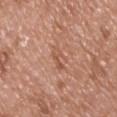Q: Was a biopsy performed?
A: total-body-photography surveillance lesion; no biopsy
Q: How was this image acquired?
A: ~15 mm crop, total-body skin-cancer survey
Q: Who is the patient?
A: male, aged 48–52
Q: Where on the body is the lesion?
A: the front of the torso